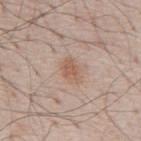Case summary:
* lighting · white-light illumination
* body site · the mid back
* subject · male, approximately 80 years of age
* acquisition · 15 mm crop, total-body photography
* lesion diameter · ≈3 mm
* image-analysis metrics · a footprint of about 4.5 mm² and an eccentricity of roughly 0.75; a border-irregularity rating of about 2.5/10 and a within-lesion color-variation index near 2/10; a classifier nevus-likeness of about 75/100 and a lesion-detection confidence of about 100/100